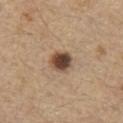Case summary:
• follow-up · no biopsy performed (imaged during a skin exam)
• illumination · white-light
• imaging modality · ~15 mm crop, total-body skin-cancer survey
• location · the chest
• lesion diameter · ≈3 mm
• image-analysis metrics · a footprint of about 6.5 mm² and a shape eccentricity near 0.4; lesion-presence confidence of about 100/100
• patient · male, roughly 70 years of age